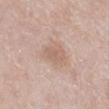follow-up — total-body-photography surveillance lesion; no biopsy